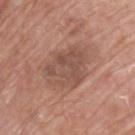Background:
An algorithmic analysis of the crop reported a border-irregularity index near 5/10, internal color variation of about 3.5 on a 0–10 scale, and a peripheral color-asymmetry measure near 1. A male patient aged approximately 65. From the upper back. A 15 mm close-up extracted from a 3D total-body photography capture. Imaged with white-light lighting. About 5 mm across.